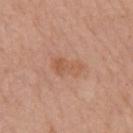Assessment: The lesion was photographed on a routine skin check and not biopsied; there is no pathology result. Image and clinical context: Located on the right upper arm. A female patient roughly 65 years of age. A region of skin cropped from a whole-body photographic capture, roughly 15 mm wide.